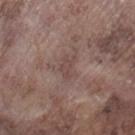Captured during whole-body skin photography for melanoma surveillance; the lesion was not biopsied. From the right lower leg. The lesion's longest dimension is about 3 mm. A male subject about 75 years old. A roughly 15 mm field-of-view crop from a total-body skin photograph.